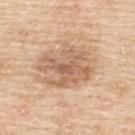The lesion was photographed on a routine skin check and not biopsied; there is no pathology result. Measured at roughly 6 mm in maximum diameter. The subject is a female approximately 55 years of age. Captured under white-light illumination. A 15 mm close-up tile from a total-body photography series done for melanoma screening. Located on the back. Automated tile analysis of the lesion measured an area of roughly 20 mm², a shape eccentricity near 0.7, and two-axis asymmetry of about 0.35. The software also gave a lesion color around L≈62 a*≈19 b*≈33 in CIELAB, roughly 11 lightness units darker than nearby skin, and a lesion-to-skin contrast of about 7 (normalized; higher = more distinct). And it measured a nevus-likeness score of about 5/100 and a detector confidence of about 100 out of 100 that the crop contains a lesion.